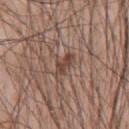Assessment: Recorded during total-body skin imaging; not selected for excision or biopsy. Context: The lesion's longest dimension is about 3 mm. An algorithmic analysis of the crop reported border irregularity of about 3.5 on a 0–10 scale, internal color variation of about 2.5 on a 0–10 scale, and radial color variation of about 1. The analysis additionally found lesion-presence confidence of about 100/100. A 15 mm crop from a total-body photograph taken for skin-cancer surveillance. The lesion is located on the chest. Imaged with white-light lighting. A male subject in their mid- to late 50s.